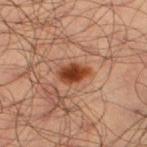Imaged during a routine full-body skin examination; the lesion was not biopsied and no histopathology is available. The patient is a male approximately 35 years of age. A lesion tile, about 15 mm wide, cut from a 3D total-body photograph. The lesion is on the left lower leg.